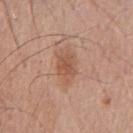Case summary:
- size · ~4 mm (longest diameter)
- acquisition · total-body-photography crop, ~15 mm field of view
- body site · the chest
- illumination · white-light illumination
- patient · male, aged around 65